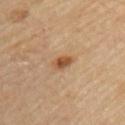follow-up=total-body-photography surveillance lesion; no biopsy
image source=total-body-photography crop, ~15 mm field of view
illumination=cross-polarized illumination
body site=the upper back
subject=male, in their mid-80s or older